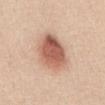Case summary:
– workup: imaged on a skin check; not biopsied